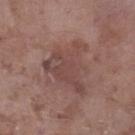Acquisition and patient details:
This image is a 15 mm lesion crop taken from a total-body photograph. The lesion is located on the leg. A male patient, in their mid- to late 70s.